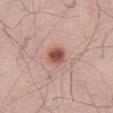Captured during whole-body skin photography for melanoma surveillance; the lesion was not biopsied. The tile uses white-light illumination. Cropped from a whole-body photographic skin survey; the tile spans about 15 mm. From the abdomen. The subject is a male about 60 years old. About 3 mm across.